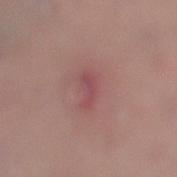| key | value |
|---|---|
| biopsy status | total-body-photography surveillance lesion; no biopsy |
| size | about 3 mm |
| subject | female, aged 33–37 |
| site | the left lower leg |
| image | total-body-photography crop, ~15 mm field of view |
| automated lesion analysis | a lesion area of about 3.5 mm², an eccentricity of roughly 0.85, and two-axis asymmetry of about 0.45; an average lesion color of about L≈48 a*≈27 b*≈18 (CIELAB), roughly 7 lightness units darker than nearby skin, and a normalized lesion–skin contrast near 5.5; a border-irregularity rating of about 4.5/10, a within-lesion color-variation index near 1/10, and peripheral color asymmetry of about 0; an automated nevus-likeness rating near 0 out of 100 and lesion-presence confidence of about 100/100 |
| illumination | white-light |